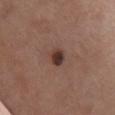Clinical impression: Imaged during a routine full-body skin examination; the lesion was not biopsied and no histopathology is available. Image and clinical context: Located on the front of the torso. A female patient approximately 60 years of age. Cropped from a total-body skin-imaging series; the visible field is about 15 mm. Captured under white-light illumination. The lesion's longest dimension is about 3 mm.